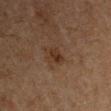Q: Is there a histopathology result?
A: imaged on a skin check; not biopsied